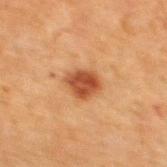Q: Is there a histopathology result?
A: catalogued during a skin exam; not biopsied
Q: Where on the body is the lesion?
A: the mid back
Q: Illumination type?
A: cross-polarized illumination
Q: Lesion size?
A: ≈3.5 mm
Q: What are the patient's age and sex?
A: male, aged 48–52
Q: What is the imaging modality?
A: 15 mm crop, total-body photography